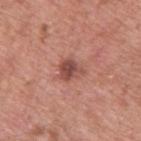Impression: Captured during whole-body skin photography for melanoma surveillance; the lesion was not biopsied. Context: A region of skin cropped from a whole-body photographic capture, roughly 15 mm wide. From the upper back. A male subject in their mid-50s.